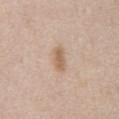Part of a total-body skin-imaging series; this lesion was reviewed on a skin check and was not flagged for biopsy. A male subject aged approximately 60. A close-up tile cropped from a whole-body skin photograph, about 15 mm across. From the abdomen. The lesion's longest dimension is about 3 mm. The lesion-visualizer software estimated a footprint of about 4.5 mm², an eccentricity of roughly 0.85, and two-axis asymmetry of about 0.2. The software also gave a lesion color around L≈61 a*≈17 b*≈31 in CIELAB and a normalized lesion–skin contrast near 7. The software also gave a border-irregularity index near 2/10, a color-variation rating of about 2/10, and a peripheral color-asymmetry measure near 0.5. And it measured a classifier nevus-likeness of about 80/100 and lesion-presence confidence of about 100/100. This is a white-light tile.